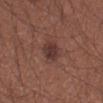<lesion>
<biopsy_status>not biopsied; imaged during a skin examination</biopsy_status>
<automated_metrics>
  <area_mm2_approx>5.0</area_mm2_approx>
  <eccentricity>0.6</eccentricity>
  <shape_asymmetry>0.25</shape_asymmetry>
  <border_irregularity_0_10>2.0</border_irregularity_0_10>
  <color_variation_0_10>3.0</color_variation_0_10>
  <peripheral_color_asymmetry>1.0</peripheral_color_asymmetry>
  <nevus_likeness_0_100>80</nevus_likeness_0_100>
  <lesion_detection_confidence_0_100>100</lesion_detection_confidence_0_100>
</automated_metrics>
<patient>
  <sex>male</sex>
  <age_approx>35</age_approx>
</patient>
<lesion_size>
  <long_diameter_mm_approx>2.5</long_diameter_mm_approx>
</lesion_size>
<image>
  <source>total-body photography crop</source>
  <field_of_view_mm>15</field_of_view_mm>
</image>
<lighting>white-light</lighting>
<site>right forearm</site>
</lesion>